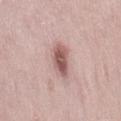notes = total-body-photography surveillance lesion; no biopsy
diameter = about 4.5 mm
illumination = white-light
body site = the back
subject = female, roughly 30 years of age
image source = ~15 mm crop, total-body skin-cancer survey